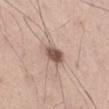Acquisition and patient details:
Located on the right thigh. The subject is a male about 55 years old. This is a white-light tile. A 15 mm close-up extracted from a 3D total-body photography capture.